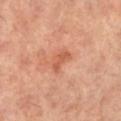The lesion was photographed on a routine skin check and not biopsied; there is no pathology result. The recorded lesion diameter is about 3 mm. The lesion is on the leg. A female patient, about 60 years old. The tile uses cross-polarized illumination. A lesion tile, about 15 mm wide, cut from a 3D total-body photograph.